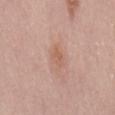Impression:
No biopsy was performed on this lesion — it was imaged during a full skin examination and was not determined to be concerning.
Context:
On the back. A female patient aged around 30. About 2.5 mm across. A 15 mm close-up extracted from a 3D total-body photography capture. An algorithmic analysis of the crop reported a footprint of about 4 mm², a shape eccentricity near 0.8, and a symmetry-axis asymmetry near 0.25. The analysis additionally found an average lesion color of about L≈60 a*≈21 b*≈29 (CIELAB), roughly 7 lightness units darker than nearby skin, and a lesion-to-skin contrast of about 5.5 (normalized; higher = more distinct). The software also gave a classifier nevus-likeness of about 0/100.